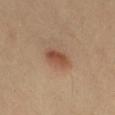– notes — imaged on a skin check; not biopsied
– diameter — ≈3 mm
– site — the leg
– patient — male, in their 40s
– image source — ~15 mm crop, total-body skin-cancer survey